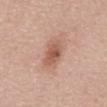Q: Is there a histopathology result?
A: no biopsy performed (imaged during a skin exam)
Q: What are the patient's age and sex?
A: male, aged approximately 70
Q: What did automated image analysis measure?
A: a lesion area of about 7.5 mm², a shape eccentricity near 0.7, and a shape-asymmetry score of about 0.2 (0 = symmetric); a lesion color around L≈56 a*≈23 b*≈29 in CIELAB and a lesion-to-skin contrast of about 7 (normalized; higher = more distinct); an automated nevus-likeness rating near 75 out of 100
Q: Lesion location?
A: the mid back
Q: What kind of image is this?
A: ~15 mm tile from a whole-body skin photo
Q: Illumination type?
A: white-light